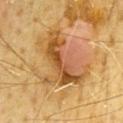follow-up: imaged on a skin check; not biopsied
image: ~15 mm tile from a whole-body skin photo
anatomic site: the chest
lesion size: ~7.5 mm (longest diameter)
subject: male, aged 63–67
image-analysis metrics: an average lesion color of about L≈56 a*≈22 b*≈43 (CIELAB), roughly 12 lightness units darker than nearby skin, and a normalized lesion–skin contrast near 8; a classifier nevus-likeness of about 0/100